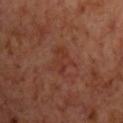* anatomic site · the chest
* acquisition · ~15 mm crop, total-body skin-cancer survey
* patient · male, aged around 70
* illumination · cross-polarized
* lesion size · about 3.5 mm